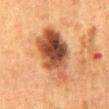Recorded during total-body skin imaging; not selected for excision or biopsy.
Longest diameter approximately 7.5 mm.
Located on the abdomen.
A 15 mm close-up extracted from a 3D total-body photography capture.
A female subject about 50 years old.